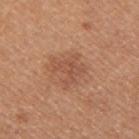Assessment: Imaged during a routine full-body skin examination; the lesion was not biopsied and no histopathology is available. Clinical summary: On the right upper arm. A male subject, roughly 30 years of age. A lesion tile, about 15 mm wide, cut from a 3D total-body photograph.